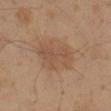Part of a total-body skin-imaging series; this lesion was reviewed on a skin check and was not flagged for biopsy. The lesion is on the right thigh. The tile uses cross-polarized illumination. Cropped from a total-body skin-imaging series; the visible field is about 15 mm. A male patient, aged 58 to 62.